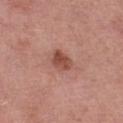Q: Is there a histopathology result?
A: catalogued during a skin exam; not biopsied
Q: What kind of image is this?
A: ~15 mm crop, total-body skin-cancer survey
Q: What is the anatomic site?
A: the left thigh
Q: Who is the patient?
A: female, aged around 70